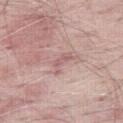Recorded during total-body skin imaging; not selected for excision or biopsy. On the right thigh. Measured at roughly 3 mm in maximum diameter. A male patient aged 63–67. The total-body-photography lesion software estimated a border-irregularity index near 6.5/10 and radial color variation of about 0. And it measured a nevus-likeness score of about 0/100. Captured under white-light illumination. Cropped from a whole-body photographic skin survey; the tile spans about 15 mm.